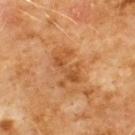Impression: No biopsy was performed on this lesion — it was imaged during a full skin examination and was not determined to be concerning. Acquisition and patient details: From the chest. A lesion tile, about 15 mm wide, cut from a 3D total-body photograph. A male patient roughly 60 years of age.